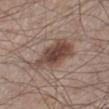Impression:
The lesion was photographed on a routine skin check and not biopsied; there is no pathology result.
Background:
A male subject aged approximately 60. An algorithmic analysis of the crop reported a footprint of about 14 mm², an outline eccentricity of about 0.9 (0 = round, 1 = elongated), and a symmetry-axis asymmetry near 0.3. The analysis additionally found a classifier nevus-likeness of about 95/100 and a detector confidence of about 100 out of 100 that the crop contains a lesion. The lesion is on the left lower leg. A region of skin cropped from a whole-body photographic capture, roughly 15 mm wide. Measured at roughly 6.5 mm in maximum diameter. This is a white-light tile.